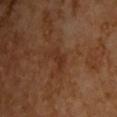The lesion was photographed on a routine skin check and not biopsied; there is no pathology result. A roughly 15 mm field-of-view crop from a total-body skin photograph. The lesion's longest dimension is about 3 mm. The tile uses cross-polarized illumination. The lesion is on the upper back. The subject is a male aged 58–62.